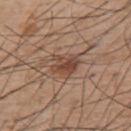Clinical impression:
The lesion was tiled from a total-body skin photograph and was not biopsied.
Context:
The lesion is located on the upper back. A male subject aged around 55. A 15 mm close-up tile from a total-body photography series done for melanoma screening.